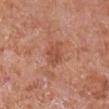Q: Is there a histopathology result?
A: catalogued during a skin exam; not biopsied
Q: What are the patient's age and sex?
A: male, aged around 65
Q: What is the imaging modality?
A: ~15 mm tile from a whole-body skin photo
Q: Illumination type?
A: white-light
Q: Lesion location?
A: the right upper arm
Q: Lesion size?
A: ≈3 mm